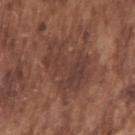* follow-up · catalogued during a skin exam; not biopsied
* automated lesion analysis · a nevus-likeness score of about 0/100
* lighting · white-light illumination
* site · the right upper arm
* diameter · about 6.5 mm
* acquisition · ~15 mm tile from a whole-body skin photo
* subject · male, about 75 years old